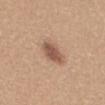biopsy status — no biopsy performed (imaged during a skin exam)